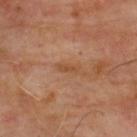The lesion was photographed on a routine skin check and not biopsied; there is no pathology result. The lesion's longest dimension is about 2.5 mm. The lesion is located on the upper back. The tile uses cross-polarized illumination. The subject is a male aged 63 to 67. A 15 mm crop from a total-body photograph taken for skin-cancer surveillance. Automated image analysis of the tile measured an area of roughly 2.5 mm² and an eccentricity of roughly 0.95. The analysis additionally found a nevus-likeness score of about 0/100 and a detector confidence of about 100 out of 100 that the crop contains a lesion.